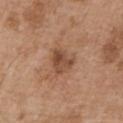<record>
  <biopsy_status>not biopsied; imaged during a skin examination</biopsy_status>
  <image>
    <source>total-body photography crop</source>
    <field_of_view_mm>15</field_of_view_mm>
  </image>
  <lesion_size>
    <long_diameter_mm_approx>3.0</long_diameter_mm_approx>
  </lesion_size>
  <site>left upper arm</site>
  <patient>
    <sex>male</sex>
    <age_approx>55</age_approx>
  </patient>
</record>